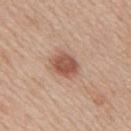Q: What are the patient's age and sex?
A: male, in their 60s
Q: How was this image acquired?
A: ~15 mm crop, total-body skin-cancer survey
Q: Lesion size?
A: ≈3.5 mm
Q: What is the anatomic site?
A: the mid back